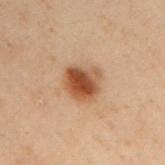follow-up: total-body-photography surveillance lesion; no biopsy | subject: male, aged 48–52 | site: the arm | illumination: cross-polarized | size: ~3.5 mm (longest diameter) | TBP lesion metrics: a shape eccentricity near 0.5 and a shape-asymmetry score of about 0.25 (0 = symmetric); about 13 CIELAB-L* units darker than the surrounding skin and a normalized lesion–skin contrast near 10.5; a classifier nevus-likeness of about 100/100 and lesion-presence confidence of about 100/100 | image: ~15 mm crop, total-body skin-cancer survey.